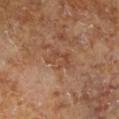image:
  source: total-body photography crop
  field_of_view_mm: 15
patient:
  sex: female
site: left lower leg
lesion_size:
  long_diameter_mm_approx: 3.5